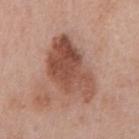Notes:
- biopsy status — catalogued during a skin exam; not biopsied
- imaging modality — total-body-photography crop, ~15 mm field of view
- body site — the mid back
- subject — male, approximately 70 years of age
- tile lighting — white-light illumination